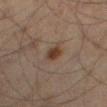Findings:
* notes · no biopsy performed (imaged during a skin exam)
* location · the right thigh
* patient · male, aged approximately 65
* image source · total-body-photography crop, ~15 mm field of view